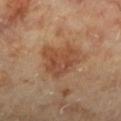This lesion was catalogued during total-body skin photography and was not selected for biopsy.
The lesion is located on the left lower leg.
A roughly 15 mm field-of-view crop from a total-body skin photograph.
The subject is a female aged 58 to 62.
Imaged with cross-polarized lighting.